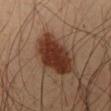Q: Was a biopsy performed?
A: catalogued during a skin exam; not biopsied
Q: Patient demographics?
A: male, roughly 35 years of age
Q: Automated lesion metrics?
A: a lesion area of about 18 mm² and an outline eccentricity of about 0.85 (0 = round, 1 = elongated); border irregularity of about 3 on a 0–10 scale, internal color variation of about 3.5 on a 0–10 scale, and radial color variation of about 1
Q: What is the anatomic site?
A: the chest
Q: How was the tile lit?
A: cross-polarized illumination
Q: What kind of image is this?
A: ~15 mm tile from a whole-body skin photo